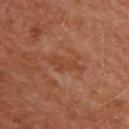Findings:
• follow-up — imaged on a skin check; not biopsied
• imaging modality — total-body-photography crop, ~15 mm field of view
• site — the upper back
• subject — male, aged 48–52